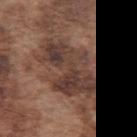illumination = white-light; image = total-body-photography crop, ~15 mm field of view; lesion diameter = ≈8 mm; location = the left upper arm; patient = male, aged 73 to 77.